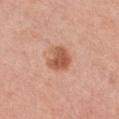Image and clinical context: This image is a 15 mm lesion crop taken from a total-body photograph. The lesion-visualizer software estimated about 12 CIELAB-L* units darker than the surrounding skin and a normalized border contrast of about 8.5. The software also gave a within-lesion color-variation index near 3/10 and a peripheral color-asymmetry measure near 1. This is a white-light tile. The lesion is located on the chest. The subject is a female aged approximately 45.